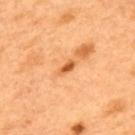The lesion was photographed on a routine skin check and not biopsied; there is no pathology result.
Automated tile analysis of the lesion measured a lesion area of about 2.5 mm², a shape eccentricity near 0.9, and a shape-asymmetry score of about 0.25 (0 = symmetric). The analysis additionally found an average lesion color of about L≈61 a*≈30 b*≈47 (CIELAB), a lesion–skin lightness drop of about 13, and a lesion-to-skin contrast of about 8.5 (normalized; higher = more distinct). It also reported a nevus-likeness score of about 20/100 and a lesion-detection confidence of about 100/100.
On the upper back.
A region of skin cropped from a whole-body photographic capture, roughly 15 mm wide.
A male subject, aged 48 to 52.
Captured under cross-polarized illumination.